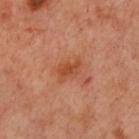Imaged during a routine full-body skin examination; the lesion was not biopsied and no histopathology is available. This is a cross-polarized tile. A close-up tile cropped from a whole-body skin photograph, about 15 mm across. The lesion is located on the chest. The subject is a female aged around 55. About 3 mm across.